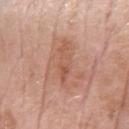Clinical impression: Captured during whole-body skin photography for melanoma surveillance; the lesion was not biopsied. Clinical summary: The recorded lesion diameter is about 6 mm. A female patient, aged approximately 70. Automated image analysis of the tile measured an area of roughly 11 mm² and a symmetry-axis asymmetry near 0.35. The analysis additionally found a mean CIELAB color near L≈57 a*≈23 b*≈30 and roughly 7 lightness units darker than nearby skin. And it measured a border-irregularity rating of about 5.5/10, a color-variation rating of about 4.5/10, and a peripheral color-asymmetry measure near 1.5. This image is a 15 mm lesion crop taken from a total-body photograph. From the arm. This is a white-light tile.